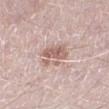Q: Is there a histopathology result?
A: catalogued during a skin exam; not biopsied
Q: Patient demographics?
A: male, approximately 50 years of age
Q: How was the tile lit?
A: white-light illumination
Q: Lesion size?
A: ~3.5 mm (longest diameter)
Q: Where on the body is the lesion?
A: the left lower leg
Q: What kind of image is this?
A: ~15 mm tile from a whole-body skin photo
Q: Automated lesion metrics?
A: a footprint of about 8 mm², a shape eccentricity near 0.5, and a symmetry-axis asymmetry near 0.25; an automated nevus-likeness rating near 15 out of 100